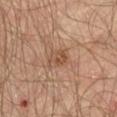Findings:
• follow-up: no biopsy performed (imaged during a skin exam)
• lesion diameter: about 2.5 mm
• tile lighting: cross-polarized illumination
• subject: male, aged approximately 65
• body site: the left thigh
• imaging modality: 15 mm crop, total-body photography
• automated lesion analysis: an area of roughly 4.5 mm² and a shape-asymmetry score of about 0.3 (0 = symmetric); an automated nevus-likeness rating near 5 out of 100 and lesion-presence confidence of about 100/100